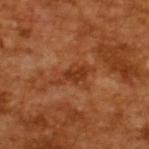biopsy status: catalogued during a skin exam; not biopsied
diameter: ≈4.5 mm
TBP lesion metrics: an area of roughly 5.5 mm² and a shape-asymmetry score of about 0.4 (0 = symmetric); an average lesion color of about L≈36 a*≈27 b*≈36 (CIELAB)
lighting: cross-polarized
patient: male, in their mid- to late 60s
acquisition: 15 mm crop, total-body photography